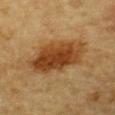Part of a total-body skin-imaging series; this lesion was reviewed on a skin check and was not flagged for biopsy.
A male subject, aged approximately 85 or older.
A lesion tile, about 15 mm wide, cut from a 3D total-body photograph.
The tile uses cross-polarized illumination.
About 8 mm across.
From the chest.